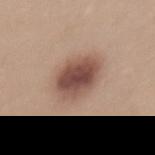Findings:
* site — the upper back
* acquisition — 15 mm crop, total-body photography
* subject — female, roughly 35 years of age
* tile lighting — white-light illumination
* lesion size — ≈5.5 mm